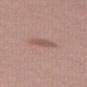<tbp_lesion>
  <biopsy_status>not biopsied; imaged during a skin examination</biopsy_status>
  <image>
    <source>total-body photography crop</source>
    <field_of_view_mm>15</field_of_view_mm>
  </image>
  <automated_metrics>
    <area_mm2_approx>3.0</area_mm2_approx>
    <eccentricity>0.85</eccentricity>
    <shape_asymmetry>0.25</shape_asymmetry>
    <border_irregularity_0_10>2.5</border_irregularity_0_10>
    <color_variation_0_10>1.0</color_variation_0_10>
    <peripheral_color_asymmetry>0.5</peripheral_color_asymmetry>
  </automated_metrics>
  <patient>
    <sex>female</sex>
    <age_approx>50</age_approx>
  </patient>
  <site>right thigh</site>
</tbp_lesion>